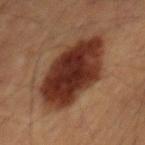Q: Is there a histopathology result?
A: imaged on a skin check; not biopsied
Q: Patient demographics?
A: male, aged approximately 65
Q: Lesion location?
A: the mid back
Q: What is the imaging modality?
A: ~15 mm tile from a whole-body skin photo